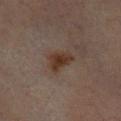Part of a total-body skin-imaging series; this lesion was reviewed on a skin check and was not flagged for biopsy. The lesion is located on the leg. Automated image analysis of the tile measured a mean CIELAB color near L≈29 a*≈14 b*≈22, about 8 CIELAB-L* units darker than the surrounding skin, and a normalized lesion–skin contrast near 9.5. And it measured border irregularity of about 3.5 on a 0–10 scale and a within-lesion color-variation index near 2.5/10. A region of skin cropped from a whole-body photographic capture, roughly 15 mm wide. A female subject aged approximately 70. Longest diameter approximately 3.5 mm. Imaged with cross-polarized lighting.